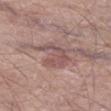The lesion was photographed on a routine skin check and not biopsied; there is no pathology result.
Located on the left thigh.
This image is a 15 mm lesion crop taken from a total-body photograph.
The subject is a male in their mid- to late 60s.
The total-body-photography lesion software estimated an area of roughly 7 mm² and an eccentricity of roughly 0.75. And it measured a lesion–skin lightness drop of about 8 and a lesion-to-skin contrast of about 6 (normalized; higher = more distinct). It also reported a border-irregularity index near 8.5/10, internal color variation of about 1.5 on a 0–10 scale, and radial color variation of about 0.5. It also reported lesion-presence confidence of about 95/100.